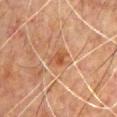Assessment:
Part of a total-body skin-imaging series; this lesion was reviewed on a skin check and was not flagged for biopsy.
Acquisition and patient details:
On the chest. A 15 mm close-up extracted from a 3D total-body photography capture. A male subject about 50 years old. Automated image analysis of the tile measured an area of roughly 4 mm², an eccentricity of roughly 0.7, and two-axis asymmetry of about 0.35. The software also gave an average lesion color of about L≈42 a*≈20 b*≈31 (CIELAB), a lesion–skin lightness drop of about 7, and a lesion-to-skin contrast of about 7 (normalized; higher = more distinct). The software also gave a classifier nevus-likeness of about 15/100. Captured under cross-polarized illumination.